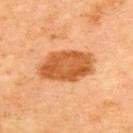Q: Was this lesion biopsied?
A: total-body-photography surveillance lesion; no biopsy
Q: What is the anatomic site?
A: the upper back
Q: What is the imaging modality?
A: total-body-photography crop, ~15 mm field of view
Q: Who is the patient?
A: female, aged around 35